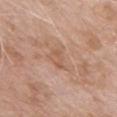biopsy status=no biopsy performed (imaged during a skin exam); image source=~15 mm crop, total-body skin-cancer survey; lighting=white-light; lesion size=≈3 mm; subject=female, roughly 75 years of age; location=the chest.